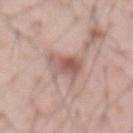Clinical impression: The lesion was photographed on a routine skin check and not biopsied; there is no pathology result. Image and clinical context: An algorithmic analysis of the crop reported a lesion area of about 8.5 mm², a shape eccentricity near 0.3, and two-axis asymmetry of about 0.4. And it measured a border-irregularity index near 5/10 and a peripheral color-asymmetry measure near 2. Cropped from a total-body skin-imaging series; the visible field is about 15 mm. A male patient, about 55 years old. Imaged with white-light lighting. Measured at roughly 4 mm in maximum diameter. The lesion is on the abdomen.